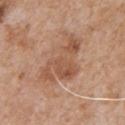Impression:
Part of a total-body skin-imaging series; this lesion was reviewed on a skin check and was not flagged for biopsy.
Acquisition and patient details:
Located on the chest. A 15 mm close-up extracted from a 3D total-body photography capture. An algorithmic analysis of the crop reported a footprint of about 15 mm², an eccentricity of roughly 0.85, and a symmetry-axis asymmetry near 0.55. And it measured border irregularity of about 7.5 on a 0–10 scale and a color-variation rating of about 4/10. The analysis additionally found a lesion-detection confidence of about 100/100. Longest diameter approximately 6.5 mm. Captured under white-light illumination. A male patient roughly 65 years of age.